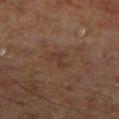  biopsy_status: not biopsied; imaged during a skin examination
  site: right lower leg
  lighting: cross-polarized
  patient:
    sex: male
    age_approx: 70
  lesion_size:
    long_diameter_mm_approx: 3.0
  image:
    source: total-body photography crop
    field_of_view_mm: 15